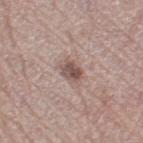Captured during whole-body skin photography for melanoma surveillance; the lesion was not biopsied. From the right thigh. The subject is a female aged 63–67. A lesion tile, about 15 mm wide, cut from a 3D total-body photograph. This is a white-light tile. Measured at roughly 2.5 mm in maximum diameter. An algorithmic analysis of the crop reported a lesion area of about 4.5 mm², an eccentricity of roughly 0.7, and a shape-asymmetry score of about 0.15 (0 = symmetric). And it measured a border-irregularity index near 1.5/10 and radial color variation of about 1.5. The software also gave an automated nevus-likeness rating near 80 out of 100.